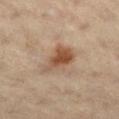On the leg. The patient is a male aged 58 to 62. Cropped from a whole-body photographic skin survey; the tile spans about 15 mm.